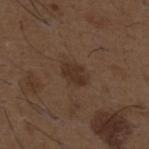Imaged during a routine full-body skin examination; the lesion was not biopsied and no histopathology is available. This image is a 15 mm lesion crop taken from a total-body photograph. The recorded lesion diameter is about 3 mm. An algorithmic analysis of the crop reported a lesion color around L≈31 a*≈15 b*≈24 in CIELAB, roughly 7 lightness units darker than nearby skin, and a normalized border contrast of about 7.5. And it measured border irregularity of about 2.5 on a 0–10 scale, a within-lesion color-variation index near 2/10, and a peripheral color-asymmetry measure near 1. The analysis additionally found a classifier nevus-likeness of about 25/100 and a detector confidence of about 100 out of 100 that the crop contains a lesion. The subject is a male roughly 50 years of age. From the back.